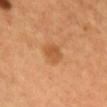Captured during whole-body skin photography for melanoma surveillance; the lesion was not biopsied. Measured at roughly 2.5 mm in maximum diameter. On the chest. An algorithmic analysis of the crop reported an automated nevus-likeness rating near 35 out of 100 and lesion-presence confidence of about 100/100. A female subject roughly 40 years of age. This is a cross-polarized tile. A lesion tile, about 15 mm wide, cut from a 3D total-body photograph.